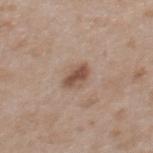{
  "biopsy_status": "not biopsied; imaged during a skin examination",
  "site": "upper back",
  "automated_metrics": {
    "vs_skin_darker_L": 12.0,
    "vs_skin_contrast_norm": 8.5
  },
  "lighting": "white-light",
  "lesion_size": {
    "long_diameter_mm_approx": 3.0
  },
  "image": {
    "source": "total-body photography crop",
    "field_of_view_mm": 15
  },
  "patient": {
    "sex": "female",
    "age_approx": 30
  }
}